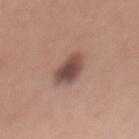The lesion was photographed on a routine skin check and not biopsied; there is no pathology result. The patient is a female in their 50s. Automated image analysis of the tile measured a footprint of about 8.5 mm², an eccentricity of roughly 0.75, and a symmetry-axis asymmetry near 0.2. The analysis additionally found a mean CIELAB color near L≈48 a*≈19 b*≈23 and about 13 CIELAB-L* units darker than the surrounding skin. And it measured a classifier nevus-likeness of about 85/100 and lesion-presence confidence of about 100/100. Imaged with white-light lighting. Cropped from a total-body skin-imaging series; the visible field is about 15 mm. The lesion is on the chest. About 4 mm across.